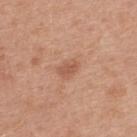Captured during whole-body skin photography for melanoma surveillance; the lesion was not biopsied.
A 15 mm close-up extracted from a 3D total-body photography capture.
The lesion is on the upper back.
A male patient, aged around 30.